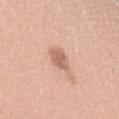This lesion was catalogued during total-body skin photography and was not selected for biopsy. The subject is a female in their mid- to late 30s. A lesion tile, about 15 mm wide, cut from a 3D total-body photograph. From the left upper arm.